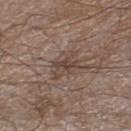Q: Was a biopsy performed?
A: no biopsy performed (imaged during a skin exam)
Q: Patient demographics?
A: male, aged around 60
Q: What is the anatomic site?
A: the right lower leg
Q: Lesion size?
A: ≈2.5 mm
Q: What is the imaging modality?
A: ~15 mm tile from a whole-body skin photo